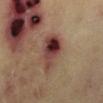Background: The tile uses cross-polarized illumination. A patient aged 58 to 62. On the left lower leg. Automated tile analysis of the lesion measured an area of roughly 12 mm², an outline eccentricity of about 0.8 (0 = round, 1 = elongated), and a symmetry-axis asymmetry near 0.3. And it measured roughly 15 lightness units darker than nearby skin and a normalized border contrast of about 12.5. A region of skin cropped from a whole-body photographic capture, roughly 15 mm wide.